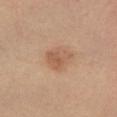Q: Was this lesion biopsied?
A: catalogued during a skin exam; not biopsied
Q: What are the patient's age and sex?
A: female, roughly 60 years of age
Q: Lesion location?
A: the leg
Q: How was the tile lit?
A: cross-polarized
Q: What kind of image is this?
A: total-body-photography crop, ~15 mm field of view